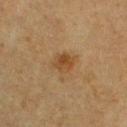workup: no biopsy performed (imaged during a skin exam) | location: the chest | illumination: cross-polarized | diameter: ~3.5 mm (longest diameter) | image source: ~15 mm tile from a whole-body skin photo | subject: male, about 75 years old | automated lesion analysis: a mean CIELAB color near L≈37 a*≈15 b*≈30, about 6 CIELAB-L* units darker than the surrounding skin, and a lesion-to-skin contrast of about 6.5 (normalized; higher = more distinct); a nevus-likeness score of about 55/100 and a detector confidence of about 100 out of 100 that the crop contains a lesion.